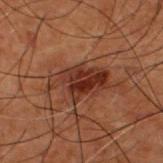image — ~15 mm crop, total-body skin-cancer survey | location — the upper back | patient — male, aged approximately 50.